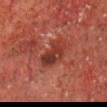This lesion was catalogued during total-body skin photography and was not selected for biopsy. Imaged with cross-polarized lighting. A male patient, in their 70s. Approximately 4 mm at its widest. A 15 mm close-up extracted from a 3D total-body photography capture. From the chest.